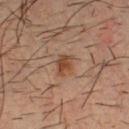The lesion was photographed on a routine skin check and not biopsied; there is no pathology result. The lesion is located on the chest. The subject is a male about 35 years old. This is a cross-polarized tile. Longest diameter approximately 2.5 mm. A region of skin cropped from a whole-body photographic capture, roughly 15 mm wide.